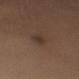{
  "biopsy_status": "not biopsied; imaged during a skin examination",
  "image": {
    "source": "total-body photography crop",
    "field_of_view_mm": 15
  },
  "site": "right upper arm",
  "patient": {
    "sex": "male",
    "age_approx": 30
  }
}